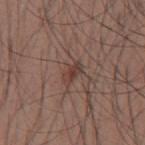notes: total-body-photography surveillance lesion; no biopsy | body site: the back | TBP lesion metrics: an area of roughly 4.5 mm² and an outline eccentricity of about 0.8 (0 = round, 1 = elongated); a lesion–skin lightness drop of about 8 and a lesion-to-skin contrast of about 6.5 (normalized; higher = more distinct); a detector confidence of about 100 out of 100 that the crop contains a lesion | subject: male, aged 33 to 37 | acquisition: 15 mm crop, total-body photography.